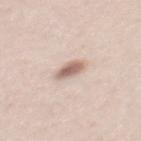Captured during whole-body skin photography for melanoma surveillance; the lesion was not biopsied. The lesion is located on the mid back. The subject is a male aged 33–37. Longest diameter approximately 3.5 mm. A 15 mm close-up extracted from a 3D total-body photography capture. Imaged with white-light lighting.